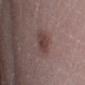The tile uses white-light illumination.
A male patient about 40 years old.
Measured at roughly 3 mm in maximum diameter.
Located on the right lower leg.
A 15 mm close-up extracted from a 3D total-body photography capture.
The lesion-visualizer software estimated a border-irregularity index near 2/10, a color-variation rating of about 3/10, and peripheral color asymmetry of about 1. And it measured an automated nevus-likeness rating near 20 out of 100.
On biopsy, histopathology showed a junctional melanocytic nevus (benign).